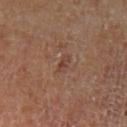<case>
<biopsy_status>not biopsied; imaged during a skin examination</biopsy_status>
<image>
  <source>total-body photography crop</source>
  <field_of_view_mm>15</field_of_view_mm>
</image>
<automated_metrics>
  <cielab_L>40</cielab_L>
  <cielab_a>20</cielab_a>
  <cielab_b>25</cielab_b>
  <vs_skin_darker_L>7.0</vs_skin_darker_L>
  <vs_skin_contrast_norm>6.0</vs_skin_contrast_norm>
  <border_irregularity_0_10>3.5</border_irregularity_0_10>
  <peripheral_color_asymmetry>0.0</peripheral_color_asymmetry>
  <nevus_likeness_0_100>0</nevus_likeness_0_100>
  <lesion_detection_confidence_0_100>100</lesion_detection_confidence_0_100>
</automated_metrics>
<site>left lower leg</site>
<lesion_size>
  <long_diameter_mm_approx>2.5</long_diameter_mm_approx>
</lesion_size>
<patient>
  <sex>male</sex>
  <age_approx>70</age_approx>
</patient>
<lighting>cross-polarized</lighting>
</case>